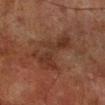Clinical impression: Recorded during total-body skin imaging; not selected for excision or biopsy. Clinical summary: A close-up tile cropped from a whole-body skin photograph, about 15 mm across. A male patient, about 70 years old. The lesion is on the right lower leg.